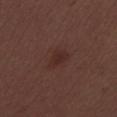biopsy_status: not biopsied; imaged during a skin examination
automated_metrics:
  vs_skin_contrast_norm: 5.5
  color_variation_0_10: 1.5
  peripheral_color_asymmetry: 0.5
patient:
  sex: male
  age_approx: 30
lighting: white-light
image:
  source: total-body photography crop
  field_of_view_mm: 15
site: mid back